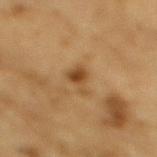Impression:
No biopsy was performed on this lesion — it was imaged during a full skin examination and was not determined to be concerning.
Image and clinical context:
A close-up tile cropped from a whole-body skin photograph, about 15 mm across. About 2.5 mm across. A male patient, aged 83 or older. This is a cross-polarized tile. From the mid back.